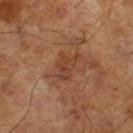Part of a total-body skin-imaging series; this lesion was reviewed on a skin check and was not flagged for biopsy.
About 4 mm across.
A 15 mm close-up extracted from a 3D total-body photography capture.
A male subject, aged around 65.
Automated image analysis of the tile measured a mean CIELAB color near L≈40 a*≈22 b*≈31, roughly 7 lightness units darker than nearby skin, and a normalized lesion–skin contrast near 6. It also reported a classifier nevus-likeness of about 0/100 and a lesion-detection confidence of about 100/100.
Imaged with cross-polarized lighting.